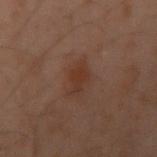image:
  source: total-body photography crop
  field_of_view_mm: 15
lighting: cross-polarized
patient:
  sex: male
  age_approx: 50
site: left arm
lesion_size:
  long_diameter_mm_approx: 3.0